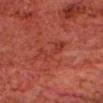Assessment: No biopsy was performed on this lesion — it was imaged during a full skin examination and was not determined to be concerning.